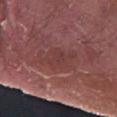notes — no biopsy performed (imaged during a skin exam) | illumination — white-light illumination | automated metrics — a mean CIELAB color near L≈39 a*≈24 b*≈21, a lesion–skin lightness drop of about 5, and a lesion-to-skin contrast of about 4.5 (normalized; higher = more distinct) | lesion size — ≈5 mm | subject — male, approximately 40 years of age | image source — total-body-photography crop, ~15 mm field of view | location — the arm.